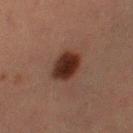{"biopsy_status": "not biopsied; imaged during a skin examination", "automated_metrics": {"area_mm2_approx": 9.5, "eccentricity": 0.35, "shape_asymmetry": 0.2, "cielab_L": 23, "cielab_a": 17, "cielab_b": 21, "vs_skin_contrast_norm": 12.5}, "lighting": "cross-polarized", "lesion_size": {"long_diameter_mm_approx": 3.5}, "patient": {"sex": "female", "age_approx": 55}, "site": "right thigh", "image": {"source": "total-body photography crop", "field_of_view_mm": 15}}